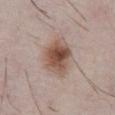{
  "biopsy_status": "not biopsied; imaged during a skin examination",
  "automated_metrics": {
    "vs_skin_darker_L": 13.0,
    "vs_skin_contrast_norm": 9.5,
    "border_irregularity_0_10": 2.0,
    "peripheral_color_asymmetry": 2.0,
    "nevus_likeness_0_100": 95,
    "lesion_detection_confidence_0_100": 100
  },
  "lesion_size": {
    "long_diameter_mm_approx": 5.0
  },
  "lighting": "white-light",
  "patient": {
    "sex": "male",
    "age_approx": 65
  },
  "site": "front of the torso",
  "image": {
    "source": "total-body photography crop",
    "field_of_view_mm": 15
  }
}